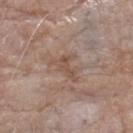Findings:
- biopsy status — no biopsy performed (imaged during a skin exam)
- lighting — white-light illumination
- subject — female, approximately 85 years of age or older
- diameter — about 4 mm
- imaging modality — ~15 mm crop, total-body skin-cancer survey
- location — the right lower leg